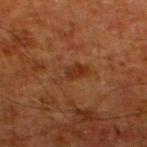Assessment: Imaged during a routine full-body skin examination; the lesion was not biopsied and no histopathology is available.